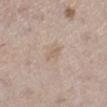The lesion was photographed on a routine skin check and not biopsied; there is no pathology result.